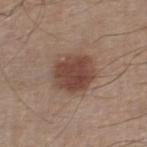Part of a total-body skin-imaging series; this lesion was reviewed on a skin check and was not flagged for biopsy.
A male patient, aged 58–62.
From the leg.
The lesion's longest dimension is about 4.5 mm.
Cropped from a total-body skin-imaging series; the visible field is about 15 mm.